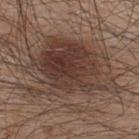Imaged during a routine full-body skin examination; the lesion was not biopsied and no histopathology is available. Captured under white-light illumination. A male patient in their mid-40s. The lesion is on the upper back. An algorithmic analysis of the crop reported an eccentricity of roughly 0.75 and a symmetry-axis asymmetry near 0.35. It also reported a mean CIELAB color near L≈38 a*≈16 b*≈23, about 11 CIELAB-L* units darker than the surrounding skin, and a lesion-to-skin contrast of about 9.5 (normalized; higher = more distinct). The analysis additionally found a color-variation rating of about 6/10 and peripheral color asymmetry of about 1.5. This image is a 15 mm lesion crop taken from a total-body photograph.